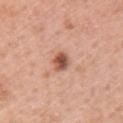The lesion was tiled from a total-body skin photograph and was not biopsied. A roughly 15 mm field-of-view crop from a total-body skin photograph. Located on the right upper arm. The patient is a male aged 58 to 62. The tile uses white-light illumination. The total-body-photography lesion software estimated a color-variation rating of about 5/10 and a peripheral color-asymmetry measure near 1.5. The software also gave a nevus-likeness score of about 90/100.